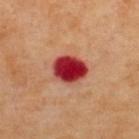Clinical impression: Recorded during total-body skin imaging; not selected for excision or biopsy. Clinical summary: Measured at roughly 4 mm in maximum diameter. A region of skin cropped from a whole-body photographic capture, roughly 15 mm wide. A male patient aged 68–72. From the upper back. This is a cross-polarized tile.